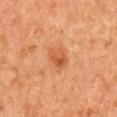biopsy status: no biopsy performed (imaged during a skin exam); patient: male, about 65 years old; imaging modality: 15 mm crop, total-body photography; diameter: about 3 mm; anatomic site: the right upper arm.